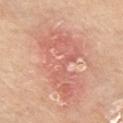Part of a total-body skin-imaging series; this lesion was reviewed on a skin check and was not flagged for biopsy.
A female subject, roughly 70 years of age.
This is a white-light tile.
A 15 mm close-up extracted from a 3D total-body photography capture.
The lesion is on the chest.
Automated image analysis of the tile measured a lesion area of about 27 mm², a shape eccentricity near 0.85, and a shape-asymmetry score of about 0.45 (0 = symmetric). The software also gave a mean CIELAB color near L≈63 a*≈27 b*≈30 and a lesion–skin lightness drop of about 8. The software also gave a border-irregularity rating of about 6.5/10, a color-variation rating of about 5/10, and radial color variation of about 1.5. The analysis additionally found a detector confidence of about 100 out of 100 that the crop contains a lesion.
About 8.5 mm across.